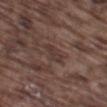{"biopsy_status": "not biopsied; imaged during a skin examination", "patient": {"sex": "male", "age_approx": 75}, "site": "left thigh", "image": {"source": "total-body photography crop", "field_of_view_mm": 15}}